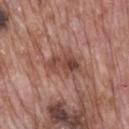| key | value |
|---|---|
| workup | imaged on a skin check; not biopsied |
| image | ~15 mm crop, total-body skin-cancer survey |
| site | the mid back |
| subject | male, approximately 70 years of age |
| lesion diameter | ~4 mm (longest diameter) |
| illumination | white-light illumination |